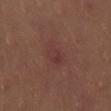| key | value |
|---|---|
| workup | catalogued during a skin exam; not biopsied |
| acquisition | total-body-photography crop, ~15 mm field of view |
| location | the right thigh |
| tile lighting | cross-polarized |
| automated metrics | a mean CIELAB color near L≈35 a*≈21 b*≈20; a classifier nevus-likeness of about 0/100 |
| patient | female, aged 33 to 37 |
| lesion size | ~4 mm (longest diameter) |